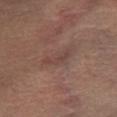Recorded during total-body skin imaging; not selected for excision or biopsy.
A male patient, roughly 45 years of age.
This is a cross-polarized tile.
A 15 mm close-up extracted from a 3D total-body photography capture.
The lesion is on the right lower leg.